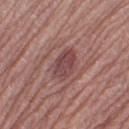Captured during whole-body skin photography for melanoma surveillance; the lesion was not biopsied. From the leg. Imaged with white-light lighting. About 4 mm across. Automated image analysis of the tile measured a border-irregularity index near 2/10 and internal color variation of about 3.5 on a 0–10 scale. A 15 mm crop from a total-body photograph taken for skin-cancer surveillance. A female subject in their 60s.